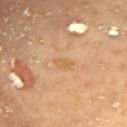follow-up: catalogued during a skin exam; not biopsied
size: about 2.5 mm
lighting: cross-polarized illumination
anatomic site: the chest
subject: female, about 60 years old
image: total-body-photography crop, ~15 mm field of view
TBP lesion metrics: a footprint of about 3.5 mm² and an eccentricity of roughly 0.75; a lesion color around L≈55 a*≈17 b*≈36 in CIELAB, about 5 CIELAB-L* units darker than the surrounding skin, and a lesion-to-skin contrast of about 4.5 (normalized; higher = more distinct); a classifier nevus-likeness of about 0/100 and lesion-presence confidence of about 100/100